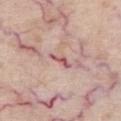Assessment: Captured during whole-body skin photography for melanoma surveillance; the lesion was not biopsied. Acquisition and patient details: From the chest. A 15 mm close-up tile from a total-body photography series done for melanoma screening. The subject is a male roughly 70 years of age. About 3 mm across.